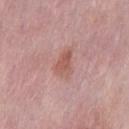Acquisition and patient details:
A close-up tile cropped from a whole-body skin photograph, about 15 mm across. Imaged with white-light lighting. The lesion is on the mid back. The lesion-visualizer software estimated a lesion area of about 6 mm², an outline eccentricity of about 0.8 (0 = round, 1 = elongated), and a symmetry-axis asymmetry near 0.4. It also reported an average lesion color of about L≈56 a*≈23 b*≈25 (CIELAB), roughly 8 lightness units darker than nearby skin, and a lesion-to-skin contrast of about 6.5 (normalized; higher = more distinct). A male subject aged 53–57. The recorded lesion diameter is about 3.5 mm.